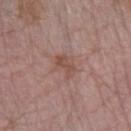workup=total-body-photography surveillance lesion; no biopsy
anatomic site=the left forearm
image=~15 mm tile from a whole-body skin photo
automated lesion analysis=an average lesion color of about L≈50 a*≈20 b*≈25 (CIELAB), about 7 CIELAB-L* units darker than the surrounding skin, and a normalized border contrast of about 6; a classifier nevus-likeness of about 0/100
tile lighting=white-light
patient=female, aged 63–67